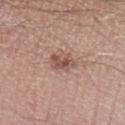| key | value |
|---|---|
| biopsy status | no biopsy performed (imaged during a skin exam) |
| anatomic site | the leg |
| subject | male, aged approximately 20 |
| image | ~15 mm tile from a whole-body skin photo |